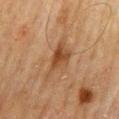Case summary:
• lighting — cross-polarized illumination
• patient — male, in their 70s
• body site — the mid back
• diameter — about 5.5 mm
• imaging modality — ~15 mm tile from a whole-body skin photo
• image-analysis metrics — a lesion area of about 9.5 mm² and an outline eccentricity of about 0.85 (0 = round, 1 = elongated); a mean CIELAB color near L≈39 a*≈18 b*≈30 and a lesion-to-skin contrast of about 7.5 (normalized; higher = more distinct); a classifier nevus-likeness of about 15/100 and a lesion-detection confidence of about 100/100